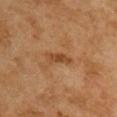Assessment:
Captured during whole-body skin photography for melanoma surveillance; the lesion was not biopsied.
Clinical summary:
The tile uses cross-polarized illumination. The recorded lesion diameter is about 3.5 mm. From the upper back. The subject is a female aged 58–62. Cropped from a whole-body photographic skin survey; the tile spans about 15 mm.